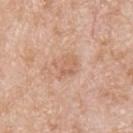| key | value |
|---|---|
| workup | catalogued during a skin exam; not biopsied |
| subject | male, aged approximately 80 |
| location | the upper back |
| acquisition | 15 mm crop, total-body photography |